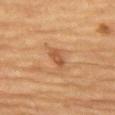{"biopsy_status": "not biopsied; imaged during a skin examination", "patient": {"sex": "male", "age_approx": "85+"}, "automated_metrics": {"cielab_L": 46, "cielab_a": 21, "cielab_b": 33, "vs_skin_darker_L": 8.0, "vs_skin_contrast_norm": 6.5}, "site": "left thigh", "image": {"source": "total-body photography crop", "field_of_view_mm": 15}, "lesion_size": {"long_diameter_mm_approx": 2.5}, "lighting": "cross-polarized"}